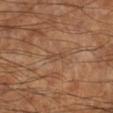{"biopsy_status": "not biopsied; imaged during a skin examination", "image": {"source": "total-body photography crop", "field_of_view_mm": 15}, "patient": {"sex": "male", "age_approx": 60}, "site": "leg"}